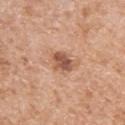acquisition: total-body-photography crop, ~15 mm field of view
lighting: white-light illumination
automated lesion analysis: a mean CIELAB color near L≈54 a*≈23 b*≈31, roughly 13 lightness units darker than nearby skin, and a lesion-to-skin contrast of about 8.5 (normalized; higher = more distinct); a border-irregularity rating of about 2/10; a nevus-likeness score of about 60/100 and a detector confidence of about 100 out of 100 that the crop contains a lesion
lesion size: ≈3 mm
body site: the left upper arm
patient: female, roughly 70 years of age